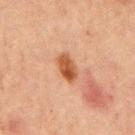Assessment: Captured during whole-body skin photography for melanoma surveillance; the lesion was not biopsied. Clinical summary: From the chest. Imaged with cross-polarized lighting. Measured at roughly 3.5 mm in maximum diameter. Cropped from a whole-body photographic skin survey; the tile spans about 15 mm. The subject is a male aged approximately 65.